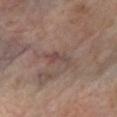Case summary:
• biopsy status — no biopsy performed (imaged during a skin exam)
• image-analysis metrics — an automated nevus-likeness rating near 0 out of 100 and a detector confidence of about 75 out of 100 that the crop contains a lesion
• body site — the leg
• illumination — cross-polarized
• acquisition — ~15 mm tile from a whole-body skin photo
• size — ≈3 mm
• patient — male, roughly 70 years of age